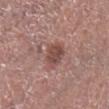Assessment: No biopsy was performed on this lesion — it was imaged during a full skin examination and was not determined to be concerning. Background: The lesion's longest dimension is about 3 mm. A male subject in their mid-50s. Captured under white-light illumination. A lesion tile, about 15 mm wide, cut from a 3D total-body photograph. The lesion is located on the right lower leg. Automated tile analysis of the lesion measured an area of roughly 7.5 mm², an eccentricity of roughly 0.45, and two-axis asymmetry of about 0.2. And it measured roughly 10 lightness units darker than nearby skin and a normalized border contrast of about 7.5. It also reported a classifier nevus-likeness of about 50/100.